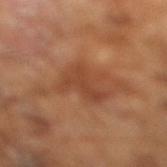notes: total-body-photography surveillance lesion; no biopsy
image-analysis metrics: an area of roughly 10 mm², an outline eccentricity of about 0.4 (0 = round, 1 = elongated), and a shape-asymmetry score of about 0.4 (0 = symmetric); a border-irregularity rating of about 5.5/10 and internal color variation of about 2 on a 0–10 scale
subject: male, in their mid- to late 60s
imaging modality: ~15 mm crop, total-body skin-cancer survey